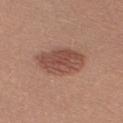Impression: Captured during whole-body skin photography for melanoma surveillance; the lesion was not biopsied. Context: Imaged with white-light lighting. On the right upper arm. A roughly 15 mm field-of-view crop from a total-body skin photograph. The patient is a female roughly 25 years of age. Longest diameter approximately 5.5 mm.